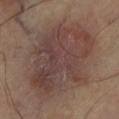<tbp_lesion>
<biopsy_status>not biopsied; imaged during a skin examination</biopsy_status>
<image>
  <source>total-body photography crop</source>
  <field_of_view_mm>15</field_of_view_mm>
</image>
<lighting>cross-polarized</lighting>
<patient>
  <sex>female</sex>
  <age_approx>60</age_approx>
</patient>
<automated_metrics>
  <area_mm2_approx>65.0</area_mm2_approx>
  <eccentricity>0.7</eccentricity>
  <shape_asymmetry>0.15</shape_asymmetry>
  <cielab_L>41</cielab_L>
  <cielab_a>16</cielab_a>
  <cielab_b>21</cielab_b>
  <vs_skin_darker_L>8.0</vs_skin_darker_L>
</automated_metrics>
<site>leg</site>
</tbp_lesion>